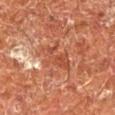biopsy status: no biopsy performed (imaged during a skin exam)
size: ≈5 mm
illumination: cross-polarized
imaging modality: total-body-photography crop, ~15 mm field of view
subject: male, in their mid- to late 60s
image-analysis metrics: a lesion–skin lightness drop of about 8 and a normalized lesion–skin contrast near 6; border irregularity of about 6.5 on a 0–10 scale, a color-variation rating of about 2/10, and radial color variation of about 0.5; an automated nevus-likeness rating near 0 out of 100 and a detector confidence of about 75 out of 100 that the crop contains a lesion
body site: the left lower leg